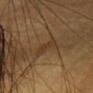  biopsy_status: not biopsied; imaged during a skin examination
  lighting: cross-polarized
  patient:
    sex: female
    age_approx: 40
  automated_metrics:
    area_mm2_approx: 3.0
    eccentricity: 0.9
    shape_asymmetry: 0.35
    cielab_L: 30
    cielab_a: 15
    cielab_b: 28
    vs_skin_darker_L: 5.0
    vs_skin_contrast_norm: 5.5
  site: head or neck
  image:
    source: total-body photography crop
    field_of_view_mm: 15
  lesion_size:
    long_diameter_mm_approx: 3.0